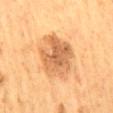Cropped from a whole-body photographic skin survey; the tile spans about 15 mm. Captured under cross-polarized illumination. The subject is a male roughly 60 years of age. The lesion's longest dimension is about 6 mm. Automated tile analysis of the lesion measured a footprint of about 21 mm², a shape eccentricity near 0.6, and a shape-asymmetry score of about 0.2 (0 = symmetric). It also reported an average lesion color of about L≈54 a*≈20 b*≈36 (CIELAB) and a normalized lesion–skin contrast near 7.5. And it measured a border-irregularity rating of about 2.5/10, a within-lesion color-variation index near 5/10, and a peripheral color-asymmetry measure near 1.5. On the mid back.